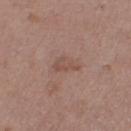Q: What lighting was used for the tile?
A: white-light
Q: What is the anatomic site?
A: the right thigh
Q: How was this image acquired?
A: ~15 mm tile from a whole-body skin photo
Q: Patient demographics?
A: male, aged 73–77
Q: Automated lesion metrics?
A: a lesion area of about 4 mm², an eccentricity of roughly 0.8, and a symmetry-axis asymmetry near 0.4
Q: How large is the lesion?
A: about 3 mm